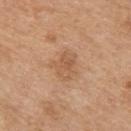No biopsy was performed on this lesion — it was imaged during a full skin examination and was not determined to be concerning.
A lesion tile, about 15 mm wide, cut from a 3D total-body photograph.
The lesion is on the arm.
About 3.5 mm across.
The patient is a male aged around 70.
Captured under white-light illumination.